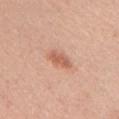Recorded during total-body skin imaging; not selected for excision or biopsy. This image is a 15 mm lesion crop taken from a total-body photograph. From the chest. The subject is a female roughly 45 years of age. The lesion-visualizer software estimated a footprint of about 5 mm², a shape eccentricity near 0.85, and a symmetry-axis asymmetry near 0.25. And it measured an average lesion color of about L≈61 a*≈25 b*≈32 (CIELAB) and roughly 11 lightness units darker than nearby skin. It also reported a border-irregularity index near 3/10, a within-lesion color-variation index near 1.5/10, and radial color variation of about 0.5. The analysis additionally found a nevus-likeness score of about 90/100 and a detector confidence of about 100 out of 100 that the crop contains a lesion. About 3.5 mm across.